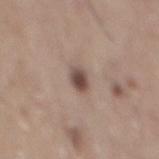- workup — total-body-photography surveillance lesion; no biopsy
- subject — male, aged 73–77
- image source — total-body-photography crop, ~15 mm field of view
- site — the back